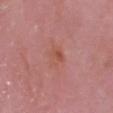Imaged during a routine full-body skin examination; the lesion was not biopsied and no histopathology is available.